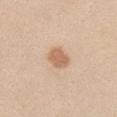{
  "biopsy_status": "not biopsied; imaged during a skin examination",
  "image": {
    "source": "total-body photography crop",
    "field_of_view_mm": 15
  },
  "automated_metrics": {
    "area_mm2_approx": 6.0,
    "eccentricity": 0.55,
    "shape_asymmetry": 0.2,
    "vs_skin_darker_L": 11.0
  },
  "patient": {
    "sex": "male",
    "age_approx": 35
  },
  "lighting": "white-light",
  "lesion_size": {
    "long_diameter_mm_approx": 3.0
  },
  "site": "right upper arm"
}